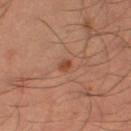Part of a total-body skin-imaging series; this lesion was reviewed on a skin check and was not flagged for biopsy. Automated tile analysis of the lesion measured an area of roughly 2 mm², an eccentricity of roughly 0.55, and a shape-asymmetry score of about 0.25 (0 = symmetric). The analysis additionally found a border-irregularity index near 2/10, internal color variation of about 1 on a 0–10 scale, and a peripheral color-asymmetry measure near 0.5. The lesion is on the leg. A region of skin cropped from a whole-body photographic capture, roughly 15 mm wide. The tile uses cross-polarized illumination. A male patient in their mid-30s.